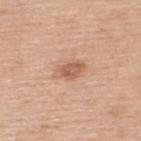  biopsy_status: not biopsied; imaged during a skin examination
  automated_metrics:
    area_mm2_approx: 5.5
    eccentricity: 0.8
    shape_asymmetry: 0.25
    cielab_L: 60
    cielab_a: 21
    cielab_b: 33
    vs_skin_darker_L: 10.0
    vs_skin_contrast_norm: 7.0
  site: upper back
  patient:
    sex: male
    age_approx: 50
  image:
    source: total-body photography crop
    field_of_view_mm: 15